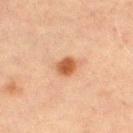Recorded during total-body skin imaging; not selected for excision or biopsy. The lesion is on the left thigh. The subject is a male approximately 70 years of age. The tile uses cross-polarized illumination. About 2.5 mm across. A lesion tile, about 15 mm wide, cut from a 3D total-body photograph.